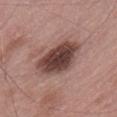{
  "biopsy_status": "not biopsied; imaged during a skin examination",
  "site": "lower back",
  "lighting": "white-light",
  "patient": {
    "sex": "male",
    "age_approx": 50
  },
  "lesion_size": {
    "long_diameter_mm_approx": 5.5
  },
  "image": {
    "source": "total-body photography crop",
    "field_of_view_mm": 15
  }
}